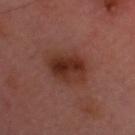Case summary:
– notes: catalogued during a skin exam; not biopsied
– patient: male, roughly 60 years of age
– tile lighting: cross-polarized
– acquisition: ~15 mm crop, total-body skin-cancer survey
– lesion size: ~5 mm (longest diameter)
– body site: the head or neck
– TBP lesion metrics: a lesion area of about 14 mm², an eccentricity of roughly 0.75, and a shape-asymmetry score of about 0.15 (0 = symmetric); a mean CIELAB color near L≈30 a*≈22 b*≈25, about 9 CIELAB-L* units darker than the surrounding skin, and a normalized border contrast of about 9; a border-irregularity rating of about 2/10, a within-lesion color-variation index near 6/10, and a peripheral color-asymmetry measure near 2.5; an automated nevus-likeness rating near 90 out of 100 and a lesion-detection confidence of about 100/100